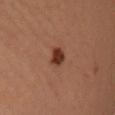follow-up = imaged on a skin check; not biopsied | lighting = cross-polarized | TBP lesion metrics = an area of roughly 4 mm², an eccentricity of roughly 0.65, and a symmetry-axis asymmetry near 0.2; a border-irregularity rating of about 2/10, internal color variation of about 2 on a 0–10 scale, and a peripheral color-asymmetry measure near 0.5; a nevus-likeness score of about 100/100 and lesion-presence confidence of about 100/100 | patient = female, about 50 years old | size = ~2.5 mm (longest diameter) | image = total-body-photography crop, ~15 mm field of view | location = the front of the torso.